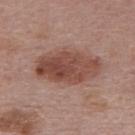Part of a total-body skin-imaging series; this lesion was reviewed on a skin check and was not flagged for biopsy. On the upper back. Imaged with white-light lighting. A female patient aged approximately 50. This image is a 15 mm lesion crop taken from a total-body photograph.